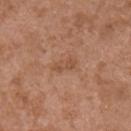  biopsy_status: not biopsied; imaged during a skin examination
  site: chest
  patient:
    sex: male
    age_approx: 75
  image:
    source: total-body photography crop
    field_of_view_mm: 15